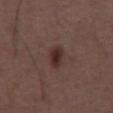| field | value |
|---|---|
| notes | catalogued during a skin exam; not biopsied |
| patient | male, in their 50s |
| image | total-body-photography crop, ~15 mm field of view |
| body site | the front of the torso |
| image-analysis metrics | a lesion area of about 4.5 mm², an outline eccentricity of about 0.8 (0 = round, 1 = elongated), and a shape-asymmetry score of about 0.25 (0 = symmetric); internal color variation of about 4 on a 0–10 scale and peripheral color asymmetry of about 1.5; an automated nevus-likeness rating near 95 out of 100 and a detector confidence of about 100 out of 100 that the crop contains a lesion |
| diameter | ~3 mm (longest diameter) |
| tile lighting | white-light illumination |